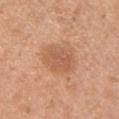{"biopsy_status": "not biopsied; imaged during a skin examination", "patient": {"sex": "female", "age_approx": 40}, "image": {"source": "total-body photography crop", "field_of_view_mm": 15}, "automated_metrics": {"area_mm2_approx": 11.0, "eccentricity": 0.75, "shape_asymmetry": 0.15, "border_irregularity_0_10": 1.5, "color_variation_0_10": 2.5, "peripheral_color_asymmetry": 1.0, "nevus_likeness_0_100": 60, "lesion_detection_confidence_0_100": 100}, "site": "chest", "lighting": "white-light", "lesion_size": {"long_diameter_mm_approx": 4.5}}